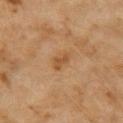Notes:
– notes · catalogued during a skin exam; not biopsied